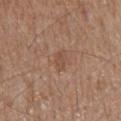biopsy status = catalogued during a skin exam; not biopsied
site = the mid back
image = ~15 mm crop, total-body skin-cancer survey
patient = male, aged 73 to 77
lighting = white-light